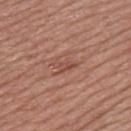Part of a total-body skin-imaging series; this lesion was reviewed on a skin check and was not flagged for biopsy. On the upper back. Measured at roughly 3 mm in maximum diameter. The tile uses white-light illumination. A female subject in their mid-50s. Automated image analysis of the tile measured a lesion area of about 3.5 mm² and a symmetry-axis asymmetry near 0.4. It also reported a lesion color around L≈49 a*≈24 b*≈28 in CIELAB and about 7 CIELAB-L* units darker than the surrounding skin. The analysis additionally found a border-irregularity rating of about 4.5/10, internal color variation of about 1 on a 0–10 scale, and peripheral color asymmetry of about 0.5. It also reported a classifier nevus-likeness of about 0/100 and a lesion-detection confidence of about 90/100. A 15 mm close-up extracted from a 3D total-body photography capture.